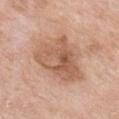workup = no biopsy performed (imaged during a skin exam) | body site = the chest | acquisition = ~15 mm tile from a whole-body skin photo | tile lighting = white-light illumination | patient = female, aged around 75.